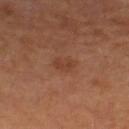The lesion was photographed on a routine skin check and not biopsied; there is no pathology result. The lesion is on the right lower leg. The tile uses cross-polarized illumination. The lesion's longest dimension is about 2.5 mm. A female patient aged approximately 65. A 15 mm close-up tile from a total-body photography series done for melanoma screening. Automated image analysis of the tile measured an area of roughly 3.5 mm² and a shape-asymmetry score of about 0.25 (0 = symmetric). And it measured a border-irregularity index near 2.5/10.